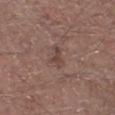Imaged with white-light lighting. The patient is a male in their mid- to late 50s. A 15 mm crop from a total-body photograph taken for skin-cancer surveillance. Measured at roughly 2.5 mm in maximum diameter. The lesion is on the leg.